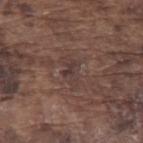workup: catalogued during a skin exam; not biopsied
subject: male, aged around 75
automated lesion analysis: a lesion area of about 5.5 mm²; an average lesion color of about L≈37 a*≈15 b*≈19 (CIELAB), a lesion–skin lightness drop of about 6, and a normalized lesion–skin contrast near 6; a border-irregularity rating of about 4/10 and a color-variation rating of about 3/10; a classifier nevus-likeness of about 0/100 and lesion-presence confidence of about 60/100
anatomic site: the left upper arm
illumination: white-light illumination
image: 15 mm crop, total-body photography
lesion size: ~3.5 mm (longest diameter)